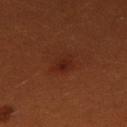<record>
<biopsy_status>not biopsied; imaged during a skin examination</biopsy_status>
<image>
  <source>total-body photography crop</source>
  <field_of_view_mm>15</field_of_view_mm>
</image>
<patient>
  <sex>female</sex>
  <age_approx>30</age_approx>
</patient>
<site>right thigh</site>
</record>